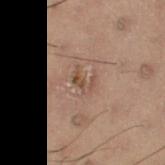Assessment:
Recorded during total-body skin imaging; not selected for excision or biopsy.
Image and clinical context:
On the left leg. Imaged with cross-polarized lighting. This image is a 15 mm lesion crop taken from a total-body photograph. Measured at roughly 2.5 mm in maximum diameter. A female patient, roughly 55 years of age. Automated tile analysis of the lesion measured a border-irregularity index near 4.5/10 and radial color variation of about 0.